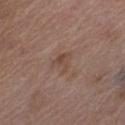Recorded during total-body skin imaging; not selected for excision or biopsy. Imaged with white-light lighting. From the leg. An algorithmic analysis of the crop reported an area of roughly 4.5 mm² and an outline eccentricity of about 0.45 (0 = round, 1 = elongated). The software also gave an average lesion color of about L≈46 a*≈17 b*≈25 (CIELAB) and a lesion-to-skin contrast of about 5.5 (normalized; higher = more distinct). It also reported an automated nevus-likeness rating near 0 out of 100 and lesion-presence confidence of about 100/100. A region of skin cropped from a whole-body photographic capture, roughly 15 mm wide. A female patient aged approximately 70.